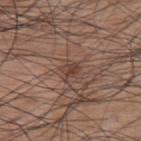Part of a total-body skin-imaging series; this lesion was reviewed on a skin check and was not flagged for biopsy. An algorithmic analysis of the crop reported a lesion area of about 4 mm² and a shape-asymmetry score of about 0.4 (0 = symmetric). And it measured a lesion color around L≈42 a*≈18 b*≈24 in CIELAB. The analysis additionally found a within-lesion color-variation index near 2.5/10. It also reported a nevus-likeness score of about 15/100 and a detector confidence of about 95 out of 100 that the crop contains a lesion. From the upper back. Measured at roughly 2.5 mm in maximum diameter. A region of skin cropped from a whole-body photographic capture, roughly 15 mm wide. The tile uses white-light illumination. The subject is a male roughly 70 years of age.